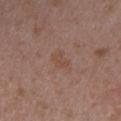Part of a total-body skin-imaging series; this lesion was reviewed on a skin check and was not flagged for biopsy.
A female subject, aged 28–32.
Located on the upper back.
Imaged with white-light lighting.
A close-up tile cropped from a whole-body skin photograph, about 15 mm across.